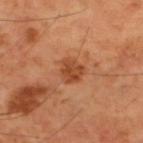The lesion was photographed on a routine skin check and not biopsied; there is no pathology result. A male subject, roughly 60 years of age. A region of skin cropped from a whole-body photographic capture, roughly 15 mm wide. Automated tile analysis of the lesion measured a lesion color around L≈45 a*≈26 b*≈36 in CIELAB, about 10 CIELAB-L* units darker than the surrounding skin, and a normalized border contrast of about 7.5. The software also gave a color-variation rating of about 3/10 and radial color variation of about 1. The software also gave a classifier nevus-likeness of about 30/100 and lesion-presence confidence of about 100/100. The lesion is on the upper back.